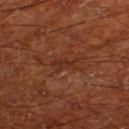<case>
<biopsy_status>not biopsied; imaged during a skin examination</biopsy_status>
<lesion_size>
  <long_diameter_mm_approx>3.0</long_diameter_mm_approx>
</lesion_size>
<site>right lower leg</site>
<lighting>cross-polarized</lighting>
<image>
  <source>total-body photography crop</source>
  <field_of_view_mm>15</field_of_view_mm>
</image>
<patient>
  <sex>male</sex>
  <age_approx>65</age_approx>
</patient>
</case>